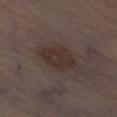{"biopsy_status": "not biopsied; imaged during a skin examination", "patient": {"sex": "male", "age_approx": 70}, "automated_metrics": {"area_mm2_approx": 13.0, "eccentricity": 0.8, "shape_asymmetry": 0.15, "border_irregularity_0_10": 2.0, "color_variation_0_10": 2.5, "peripheral_color_asymmetry": 1.0}, "site": "leg", "lighting": "white-light", "image": {"source": "total-body photography crop", "field_of_view_mm": 15}, "lesion_size": {"long_diameter_mm_approx": 5.5}}